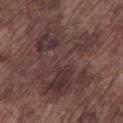workup: catalogued during a skin exam; not biopsied
illumination: white-light
subject: male, aged approximately 75
imaging modality: total-body-photography crop, ~15 mm field of view
automated lesion analysis: a lesion area of about 50 mm² and an eccentricity of roughly 0.6; a mean CIELAB color near L≈35 a*≈17 b*≈17, roughly 7 lightness units darker than nearby skin, and a lesion-to-skin contrast of about 7 (normalized; higher = more distinct); a color-variation rating of about 6/10; an automated nevus-likeness rating near 0 out of 100 and lesion-presence confidence of about 90/100
body site: the left lower leg